A 15 mm close-up tile from a total-body photography series done for melanoma screening, a male patient in their mid-60s, the lesion is on the head or neck.
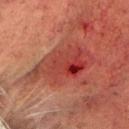Findings:
– pathology: a nodular basal cell carcinoma (malignant)